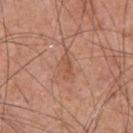This lesion was catalogued during total-body skin photography and was not selected for biopsy.
On the chest.
A male patient roughly 55 years of age.
Cropped from a total-body skin-imaging series; the visible field is about 15 mm.